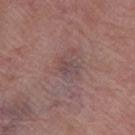| field | value |
|---|---|
| notes | catalogued during a skin exam; not biopsied |
| illumination | white-light illumination |
| diameter | ≈2.5 mm |
| subject | female, aged around 70 |
| image source | ~15 mm tile from a whole-body skin photo |
| body site | the left thigh |